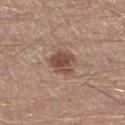Findings:
– workup: catalogued during a skin exam; not biopsied
– body site: the leg
– subject: male, aged approximately 30
– image source: ~15 mm tile from a whole-body skin photo